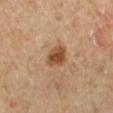Case summary:
- follow-up · catalogued during a skin exam; not biopsied
- image source · total-body-photography crop, ~15 mm field of view
- location · the left lower leg
- subject · male, in their mid- to late 60s
- TBP lesion metrics · a lesion area of about 6.5 mm², an outline eccentricity of about 0.7 (0 = round, 1 = elongated), and a symmetry-axis asymmetry near 0.2; an average lesion color of about L≈49 a*≈21 b*≈34 (CIELAB), roughly 13 lightness units darker than nearby skin, and a lesion-to-skin contrast of about 9.5 (normalized; higher = more distinct)
- lesion diameter · ≈3.5 mm